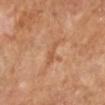  biopsy_status: not biopsied; imaged during a skin examination
  image:
    source: total-body photography crop
    field_of_view_mm: 15
  patient:
    sex: female
    age_approx: 65
  site: right lower leg